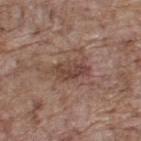Clinical impression:
Part of a total-body skin-imaging series; this lesion was reviewed on a skin check and was not flagged for biopsy.
Background:
The lesion is on the upper back. A male subject, aged around 70. This is a white-light tile. Cropped from a total-body skin-imaging series; the visible field is about 15 mm.